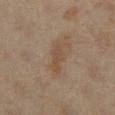{"biopsy_status": "not biopsied; imaged during a skin examination", "image": {"source": "total-body photography crop", "field_of_view_mm": 15}, "lesion_size": {"long_diameter_mm_approx": 3.5}, "patient": {"sex": "female", "age_approx": 40}, "lighting": "cross-polarized", "site": "right lower leg", "automated_metrics": {"area_mm2_approx": 4.0, "eccentricity": 0.9, "shape_asymmetry": 0.35, "nevus_likeness_0_100": 0, "lesion_detection_confidence_0_100": 100}}